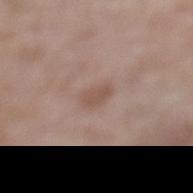{
  "site": "left lower leg",
  "patient": {
    "sex": "female",
    "age_approx": 75
  },
  "image": {
    "source": "total-body photography crop",
    "field_of_view_mm": 15
  },
  "lesion_size": {
    "long_diameter_mm_approx": 3.0
  },
  "lighting": "white-light"
}